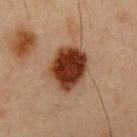No biopsy was performed on this lesion — it was imaged during a full skin examination and was not determined to be concerning.
The tile uses cross-polarized illumination.
A male patient aged 53 to 57.
The lesion is on the chest.
A 15 mm close-up extracted from a 3D total-body photography capture.
The lesion-visualizer software estimated a lesion–skin lightness drop of about 18. The software also gave a border-irregularity index near 2/10, a within-lesion color-variation index near 4/10, and radial color variation of about 1. The analysis additionally found a detector confidence of about 100 out of 100 that the crop contains a lesion.
The recorded lesion diameter is about 5 mm.